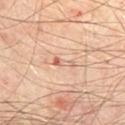<lesion>
<biopsy_status>not biopsied; imaged during a skin examination</biopsy_status>
<patient>
  <sex>male</sex>
  <age_approx>65</age_approx>
</patient>
<image>
  <source>total-body photography crop</source>
  <field_of_view_mm>15</field_of_view_mm>
</image>
<site>abdomen</site>
</lesion>